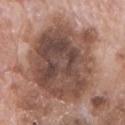This lesion was catalogued during total-body skin photography and was not selected for biopsy. Imaged with white-light lighting. A roughly 15 mm field-of-view crop from a total-body skin photograph. The subject is a male approximately 70 years of age. Located on the chest.